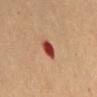Notes:
* biopsy status · catalogued during a skin exam; not biopsied
* location · the mid back
* patient · female, aged 68–72
* acquisition · total-body-photography crop, ~15 mm field of view
* diameter · ≈2.5 mm
* image-analysis metrics · a lesion area of about 4.5 mm², an eccentricity of roughly 0.7, and a shape-asymmetry score of about 0.2 (0 = symmetric); a color-variation rating of about 3/10 and radial color variation of about 0.5; an automated nevus-likeness rating near 0 out of 100 and lesion-presence confidence of about 100/100